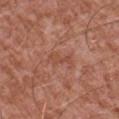| key | value |
|---|---|
| follow-up | total-body-photography surveillance lesion; no biopsy |
| subject | male, aged around 45 |
| body site | the front of the torso |
| image source | ~15 mm crop, total-body skin-cancer survey |